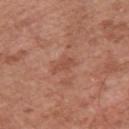| field | value |
|---|---|
| follow-up | catalogued during a skin exam; not biopsied |
| location | the left upper arm |
| image source | total-body-photography crop, ~15 mm field of view |
| size | about 2.5 mm |
| TBP lesion metrics | an area of roughly 3.5 mm², an eccentricity of roughly 0.8, and a symmetry-axis asymmetry near 0.25; a border-irregularity index near 3/10, a within-lesion color-variation index near 1/10, and peripheral color asymmetry of about 0.5; an automated nevus-likeness rating near 0 out of 100 and lesion-presence confidence of about 100/100 |
| subject | male, roughly 65 years of age |
| tile lighting | white-light illumination |